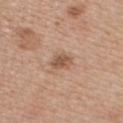Captured during whole-body skin photography for melanoma surveillance; the lesion was not biopsied.